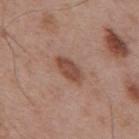Case summary:
- workup · total-body-photography surveillance lesion; no biopsy
- lesion diameter · about 4 mm
- image source · total-body-photography crop, ~15 mm field of view
- tile lighting · white-light illumination
- body site · the mid back
- automated metrics · a footprint of about 6.5 mm², a shape eccentricity near 0.85, and a shape-asymmetry score of about 0.2 (0 = symmetric); a lesion color around L≈48 a*≈21 b*≈28 in CIELAB, roughly 10 lightness units darker than nearby skin, and a lesion-to-skin contrast of about 8 (normalized; higher = more distinct); border irregularity of about 2 on a 0–10 scale, a color-variation rating of about 2.5/10, and peripheral color asymmetry of about 1; a nevus-likeness score of about 80/100 and a lesion-detection confidence of about 100/100
- subject · male, approximately 55 years of age